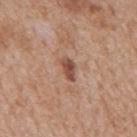biopsy status=total-body-photography surveillance lesion; no biopsy
size=~3 mm (longest diameter)
acquisition=15 mm crop, total-body photography
patient=male, approximately 65 years of age
automated metrics=a lesion area of about 4 mm², a shape eccentricity near 0.85, and two-axis asymmetry of about 0.2; a border-irregularity rating of about 2/10, internal color variation of about 3.5 on a 0–10 scale, and a peripheral color-asymmetry measure near 1
body site=the mid back
tile lighting=white-light illumination